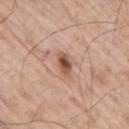The patient is a male approximately 70 years of age. Captured under white-light illumination. The lesion is on the right upper arm. Cropped from a total-body skin-imaging series; the visible field is about 15 mm. About 3 mm across.